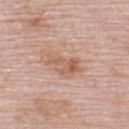The lesion was tiled from a total-body skin photograph and was not biopsied. The total-body-photography lesion software estimated a footprint of about 8 mm², an outline eccentricity of about 0.85 (0 = round, 1 = elongated), and a shape-asymmetry score of about 0.3 (0 = symmetric). The analysis additionally found a lesion color around L≈60 a*≈21 b*≈30 in CIELAB, a lesion–skin lightness drop of about 9, and a normalized lesion–skin contrast near 6.5. It also reported an automated nevus-likeness rating near 35 out of 100 and a detector confidence of about 100 out of 100 that the crop contains a lesion. From the upper back. A region of skin cropped from a whole-body photographic capture, roughly 15 mm wide. The subject is a female aged around 65. Measured at roughly 4.5 mm in maximum diameter. Imaged with white-light lighting.